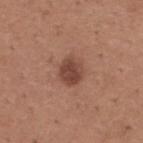Q: What is the anatomic site?
A: the upper back
Q: Who is the patient?
A: male, approximately 65 years of age
Q: What is the imaging modality?
A: ~15 mm crop, total-body skin-cancer survey
Q: How was the tile lit?
A: white-light